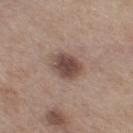workup: no biopsy performed (imaged during a skin exam); patient: female, aged 53 to 57; image source: ~15 mm crop, total-body skin-cancer survey; body site: the right thigh.